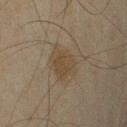This lesion was catalogued during total-body skin photography and was not selected for biopsy.
A male patient aged 63–67.
An algorithmic analysis of the crop reported a border-irregularity index near 3/10, a color-variation rating of about 2/10, and a peripheral color-asymmetry measure near 0.5.
This is a cross-polarized tile.
Located on the right upper arm.
The lesion's longest dimension is about 4 mm.
A lesion tile, about 15 mm wide, cut from a 3D total-body photograph.